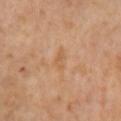| feature | finding |
|---|---|
| workup | catalogued during a skin exam; not biopsied |
| subject | female, roughly 55 years of age |
| location | the right upper arm |
| size | ~2.5 mm (longest diameter) |
| image | ~15 mm tile from a whole-body skin photo |